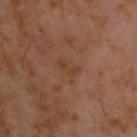No biopsy was performed on this lesion — it was imaged during a full skin examination and was not determined to be concerning. From the back. Longest diameter approximately 2.5 mm. A roughly 15 mm field-of-view crop from a total-body skin photograph. A male patient, roughly 60 years of age. Captured under cross-polarized illumination.